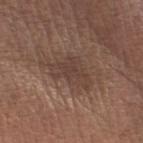Clinical impression: Part of a total-body skin-imaging series; this lesion was reviewed on a skin check and was not flagged for biopsy. Background: On the leg. A female patient about 65 years old. Imaged with white-light lighting. An algorithmic analysis of the crop reported an average lesion color of about L≈40 a*≈16 b*≈23 (CIELAB), about 7 CIELAB-L* units darker than the surrounding skin, and a normalized lesion–skin contrast near 6. Approximately 5 mm at its widest. Cropped from a whole-body photographic skin survey; the tile spans about 15 mm.